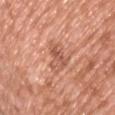Recorded during total-body skin imaging; not selected for excision or biopsy. Located on the chest. A roughly 15 mm field-of-view crop from a total-body skin photograph. Captured under white-light illumination. The subject is a male roughly 45 years of age.